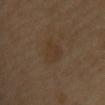– notes · total-body-photography surveillance lesion; no biopsy
– acquisition · ~15 mm tile from a whole-body skin photo
– body site · the chest
– diameter · ≈3 mm
– tile lighting · cross-polarized illumination
– subject · male, aged 38–42
– image-analysis metrics · a border-irregularity rating of about 3/10, a within-lesion color-variation index near 2/10, and a peripheral color-asymmetry measure near 0.5